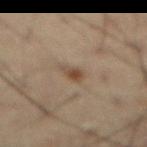lesion diameter — about 2.5 mm; lighting — cross-polarized illumination; patient — male, aged 58 to 62; imaging modality — 15 mm crop, total-body photography.